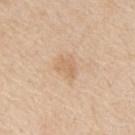Imaged during a routine full-body skin examination; the lesion was not biopsied and no histopathology is available.
The lesion is located on the mid back.
A region of skin cropped from a whole-body photographic capture, roughly 15 mm wide.
A male patient roughly 60 years of age.
Automated tile analysis of the lesion measured a lesion color around L≈66 a*≈18 b*≈35 in CIELAB and a lesion–skin lightness drop of about 7. The software also gave a color-variation rating of about 1.5/10 and peripheral color asymmetry of about 0.5. The analysis additionally found a classifier nevus-likeness of about 0/100 and a lesion-detection confidence of about 100/100.
Imaged with white-light lighting.